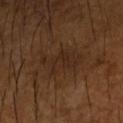Impression: Part of a total-body skin-imaging series; this lesion was reviewed on a skin check and was not flagged for biopsy. Image and clinical context: The tile uses cross-polarized illumination. A region of skin cropped from a whole-body photographic capture, roughly 15 mm wide. The total-body-photography lesion software estimated about 5 CIELAB-L* units darker than the surrounding skin. It also reported a border-irregularity index near 5/10, a within-lesion color-variation index near 2/10, and peripheral color asymmetry of about 0.5. The lesion is on the right forearm. A male subject roughly 65 years of age.